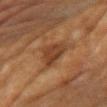Captured during whole-body skin photography for melanoma surveillance; the lesion was not biopsied. The lesion is located on the front of the torso. A 15 mm crop from a total-body photograph taken for skin-cancer surveillance. A female subject, roughly 80 years of age.